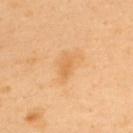Case summary:
- workup: no biopsy performed (imaged during a skin exam)
- anatomic site: the upper back
- image: total-body-photography crop, ~15 mm field of view
- size: ~2.5 mm (longest diameter)
- automated metrics: a border-irregularity rating of about 2.5/10 and internal color variation of about 1 on a 0–10 scale; an automated nevus-likeness rating near 0 out of 100 and a lesion-detection confidence of about 100/100
- illumination: cross-polarized
- subject: male, aged 38 to 42